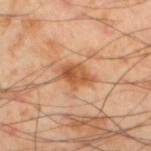<record>
<biopsy_status>not biopsied; imaged during a skin examination</biopsy_status>
<patient>
  <sex>male</sex>
  <age_approx>55</age_approx>
</patient>
<lighting>cross-polarized</lighting>
<lesion_size>
  <long_diameter_mm_approx>4.0</long_diameter_mm_approx>
</lesion_size>
<image>
  <source>total-body photography crop</source>
  <field_of_view_mm>15</field_of_view_mm>
</image>
<site>left lower leg</site>
</record>